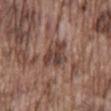This lesion was catalogued during total-body skin photography and was not selected for biopsy. The subject is a male about 75 years old. Located on the back. A region of skin cropped from a whole-body photographic capture, roughly 15 mm wide.